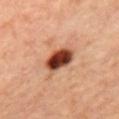Findings:
- biopsy status — no biopsy performed (imaged during a skin exam)
- tile lighting — cross-polarized
- acquisition — ~15 mm tile from a whole-body skin photo
- body site — the back
- subject — female, aged approximately 45
- diameter — ~4 mm (longest diameter)